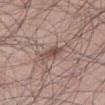Assessment: The lesion was photographed on a routine skin check and not biopsied; there is no pathology result. Background: On the right thigh. A 15 mm close-up extracted from a 3D total-body photography capture. The subject is a male in their mid- to late 40s.